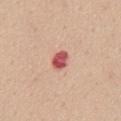Q: Was a biopsy performed?
A: total-body-photography surveillance lesion; no biopsy
Q: What is the anatomic site?
A: the chest
Q: What is the lesion's diameter?
A: ≈3 mm
Q: Illumination type?
A: white-light illumination
Q: Patient demographics?
A: female, aged around 45
Q: What kind of image is this?
A: 15 mm crop, total-body photography